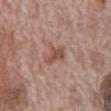Recorded during total-body skin imaging; not selected for excision or biopsy. A male patient, roughly 70 years of age. A 15 mm close-up extracted from a 3D total-body photography capture. Imaged with white-light lighting. Measured at roughly 3 mm in maximum diameter. The lesion is located on the abdomen. The total-body-photography lesion software estimated a footprint of about 5 mm² and an outline eccentricity of about 0.8 (0 = round, 1 = elongated). The analysis additionally found border irregularity of about 3.5 on a 0–10 scale, a color-variation rating of about 2/10, and radial color variation of about 0.5. The analysis additionally found an automated nevus-likeness rating near 0 out of 100 and a lesion-detection confidence of about 100/100.